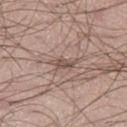Case summary:
– notes — imaged on a skin check; not biopsied
– location — the left thigh
– imaging modality — total-body-photography crop, ~15 mm field of view
– subject — male, roughly 20 years of age
– automated metrics — a lesion area of about 3 mm² and an eccentricity of roughly 0.85; a lesion–skin lightness drop of about 10 and a lesion-to-skin contrast of about 7 (normalized; higher = more distinct); an automated nevus-likeness rating near 0 out of 100 and lesion-presence confidence of about 65/100
– lighting — white-light